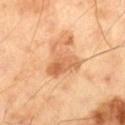follow-up: catalogued during a skin exam; not biopsied
subject: male, approximately 70 years of age
tile lighting: cross-polarized illumination
anatomic site: the left thigh
acquisition: total-body-photography crop, ~15 mm field of view